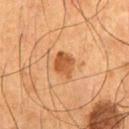site: the front of the torso | lesion size: ≈3.5 mm | patient: male, aged approximately 55 | lighting: cross-polarized | imaging modality: 15 mm crop, total-body photography.